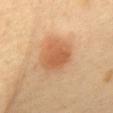Captured during whole-body skin photography for melanoma surveillance; the lesion was not biopsied. Automated image analysis of the tile measured a lesion color around L≈58 a*≈24 b*≈38 in CIELAB, a lesion–skin lightness drop of about 10, and a normalized border contrast of about 7. It also reported border irregularity of about 2 on a 0–10 scale, internal color variation of about 3 on a 0–10 scale, and a peripheral color-asymmetry measure near 1. It also reported a classifier nevus-likeness of about 100/100 and a detector confidence of about 100 out of 100 that the crop contains a lesion. This image is a 15 mm lesion crop taken from a total-body photograph. The tile uses cross-polarized illumination. Measured at roughly 4 mm in maximum diameter. The lesion is located on the abdomen. A patient aged around 60.